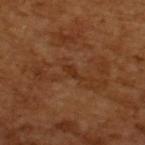biopsy status = no biopsy performed (imaged during a skin exam) | subject = male, aged approximately 65 | image source = total-body-photography crop, ~15 mm field of view.